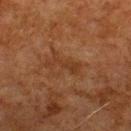workup: imaged on a skin check; not biopsied | patient: male, aged 78 to 82 | site: the back | acquisition: ~15 mm tile from a whole-body skin photo.